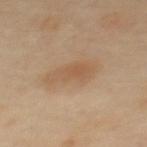| key | value |
|---|---|
| workup | catalogued during a skin exam; not biopsied |
| site | the upper back |
| subject | female, approximately 60 years of age |
| automated metrics | a footprint of about 10 mm² and an outline eccentricity of about 0.9 (0 = round, 1 = elongated); a lesion color around L≈57 a*≈17 b*≈34 in CIELAB and roughly 7 lightness units darker than nearby skin |
| acquisition | total-body-photography crop, ~15 mm field of view |
| lighting | cross-polarized |
| lesion diameter | about 5.5 mm |